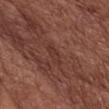Findings:
– biopsy status: no biopsy performed (imaged during a skin exam)
– illumination: white-light illumination
– patient: female, in their 80s
– lesion diameter: ≈3 mm
– TBP lesion metrics: border irregularity of about 3 on a 0–10 scale and radial color variation of about 0.5
– site: the chest
– imaging modality: ~15 mm crop, total-body skin-cancer survey